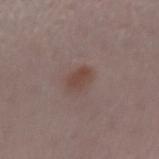- notes — no biopsy performed (imaged during a skin exam)
- automated metrics — a border-irregularity rating of about 2/10 and a within-lesion color-variation index near 2/10; a nevus-likeness score of about 70/100 and a detector confidence of about 100 out of 100 that the crop contains a lesion
- patient — female, approximately 45 years of age
- site — the right forearm
- imaging modality — ~15 mm tile from a whole-body skin photo